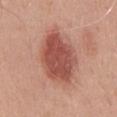notes=total-body-photography surveillance lesion; no biopsy
TBP lesion metrics=an automated nevus-likeness rating near 100 out of 100 and a lesion-detection confidence of about 100/100
imaging modality=~15 mm tile from a whole-body skin photo
patient=male, aged 48 to 52
location=the chest